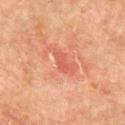Clinical impression: This lesion was catalogued during total-body skin photography and was not selected for biopsy. Image and clinical context: The tile uses cross-polarized illumination. Longest diameter approximately 2.5 mm. The lesion-visualizer software estimated an area of roughly 3 mm², an outline eccentricity of about 0.9 (0 = round, 1 = elongated), and a symmetry-axis asymmetry near 0.25. The software also gave border irregularity of about 3 on a 0–10 scale, internal color variation of about 0 on a 0–10 scale, and peripheral color asymmetry of about 0. A male patient roughly 75 years of age. The lesion is on the chest. A 15 mm close-up extracted from a 3D total-body photography capture.